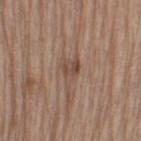Findings:
* biopsy status: catalogued during a skin exam; not biopsied
* imaging modality: ~15 mm tile from a whole-body skin photo
* patient: male, in their 70s
* illumination: white-light illumination
* size: about 3.5 mm
* site: the left thigh